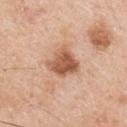Clinical impression:
Imaged during a routine full-body skin examination; the lesion was not biopsied and no histopathology is available.